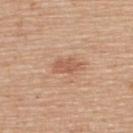Clinical impression: Recorded during total-body skin imaging; not selected for excision or biopsy. Image and clinical context: The lesion is located on the upper back. The total-body-photography lesion software estimated an average lesion color of about L≈57 a*≈23 b*≈32 (CIELAB), about 9 CIELAB-L* units darker than the surrounding skin, and a normalized lesion–skin contrast near 6. And it measured a classifier nevus-likeness of about 25/100 and lesion-presence confidence of about 100/100. The patient is a male roughly 55 years of age. Captured under white-light illumination. Longest diameter approximately 3 mm. A 15 mm close-up extracted from a 3D total-body photography capture.